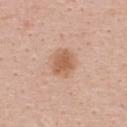{"biopsy_status": "not biopsied; imaged during a skin examination", "patient": {"sex": "female", "age_approx": 35}, "image": {"source": "total-body photography crop", "field_of_view_mm": 15}, "lighting": "white-light", "site": "upper back", "lesion_size": {"long_diameter_mm_approx": 4.0}}